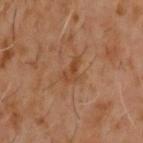Captured during whole-body skin photography for melanoma surveillance; the lesion was not biopsied. From the upper back. This is a cross-polarized tile. The recorded lesion diameter is about 3 mm. This image is a 15 mm lesion crop taken from a total-body photograph. Automated tile analysis of the lesion measured a mean CIELAB color near L≈42 a*≈20 b*≈32, about 6 CIELAB-L* units darker than the surrounding skin, and a normalized lesion–skin contrast near 6. The software also gave a color-variation rating of about 1.5/10. The software also gave a nevus-likeness score of about 0/100. A male subject, aged 58–62.